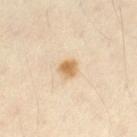notes: imaged on a skin check; not biopsied
subject: male, aged 28 to 32
lighting: cross-polarized
body site: the leg
acquisition: total-body-photography crop, ~15 mm field of view